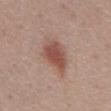Q: Was a biopsy performed?
A: imaged on a skin check; not biopsied
Q: Who is the patient?
A: male, approximately 55 years of age
Q: Illumination type?
A: white-light
Q: What is the lesion's diameter?
A: about 4.5 mm
Q: What is the imaging modality?
A: ~15 mm crop, total-body skin-cancer survey
Q: What is the anatomic site?
A: the abdomen